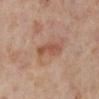The patient is a female roughly 55 years of age. Located on the right lower leg. A 15 mm crop from a total-body photograph taken for skin-cancer surveillance.